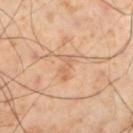No biopsy was performed on this lesion — it was imaged during a full skin examination and was not determined to be concerning. The tile uses cross-polarized illumination. Approximately 2.5 mm at its widest. A 15 mm close-up extracted from a 3D total-body photography capture. The patient is a male aged 53–57. The lesion is on the left lower leg.